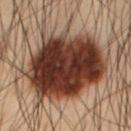<record>
<biopsy_status>not biopsied; imaged during a skin examination</biopsy_status>
<automated_metrics>
  <eccentricity>0.6</eccentricity>
  <shape_asymmetry>0.15</shape_asymmetry>
  <cielab_L>29</cielab_L>
  <cielab_a>17</cielab_a>
  <cielab_b>22</cielab_b>
  <vs_skin_darker_L>18.0</vs_skin_darker_L>
  <vs_skin_contrast_norm>16.5</vs_skin_contrast_norm>
</automated_metrics>
<site>front of the torso</site>
<lesion_size>
  <long_diameter_mm_approx>10.5</long_diameter_mm_approx>
</lesion_size>
<image>
  <source>total-body photography crop</source>
  <field_of_view_mm>15</field_of_view_mm>
</image>
<patient>
  <sex>male</sex>
  <age_approx>55</age_approx>
</patient>
<lighting>cross-polarized</lighting>
</record>